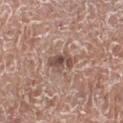Captured during whole-body skin photography for melanoma surveillance; the lesion was not biopsied. A male patient in their mid- to late 70s. The total-body-photography lesion software estimated an average lesion color of about L≈50 a*≈18 b*≈24 (CIELAB), roughly 11 lightness units darker than nearby skin, and a normalized lesion–skin contrast near 8. The software also gave a border-irregularity index near 4/10, a color-variation rating of about 4.5/10, and a peripheral color-asymmetry measure near 1.5. This is a white-light tile. The recorded lesion diameter is about 3.5 mm. Cropped from a total-body skin-imaging series; the visible field is about 15 mm. Located on the right lower leg.